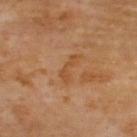anatomic site — the upper back | lesion diameter — about 3.5 mm | subject — male, aged 68 to 72 | illumination — cross-polarized | image — ~15 mm tile from a whole-body skin photo.